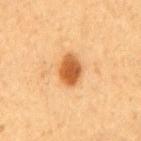Case summary:
• workup · catalogued during a skin exam; not biopsied